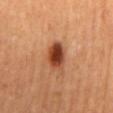Context: Cropped from a total-body skin-imaging series; the visible field is about 15 mm. The lesion's longest dimension is about 3.5 mm. On the mid back. The subject is a male roughly 70 years of age.